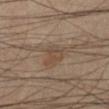workup: imaged on a skin check; not biopsied | site: the left lower leg | subject: male, aged 53 to 57 | lighting: cross-polarized illumination | image source: 15 mm crop, total-body photography | lesion size: ≈2.5 mm.